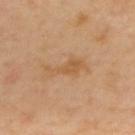The lesion was photographed on a routine skin check and not biopsied; there is no pathology result. This image is a 15 mm lesion crop taken from a total-body photograph. The total-body-photography lesion software estimated an area of roughly 6 mm², a shape eccentricity near 0.95, and two-axis asymmetry of about 0.5. The software also gave a mean CIELAB color near L≈56 a*≈19 b*≈38 and a lesion-to-skin contrast of about 5.5 (normalized; higher = more distinct). It also reported a border-irregularity rating of about 6.5/10, a color-variation rating of about 1.5/10, and a peripheral color-asymmetry measure near 0.5. The analysis additionally found a nevus-likeness score of about 0/100 and lesion-presence confidence of about 100/100. The patient is a male in their mid-60s. The lesion is on the upper back. Captured under cross-polarized illumination. The lesion's longest dimension is about 5 mm.